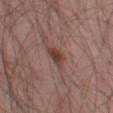  biopsy_status: not biopsied; imaged during a skin examination
  image:
    source: total-body photography crop
    field_of_view_mm: 15
  site: left thigh
  automated_metrics:
    eccentricity: 0.85
    border_irregularity_0_10: 3.0
    peripheral_color_asymmetry: 0.5
    nevus_likeness_0_100: 80
    lesion_detection_confidence_0_100: 100
  patient:
    sex: male
    age_approx: 55
  lighting: white-light
  lesion_size:
    long_diameter_mm_approx: 3.0